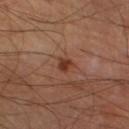Assessment:
This lesion was catalogued during total-body skin photography and was not selected for biopsy.
Clinical summary:
On the leg. A region of skin cropped from a whole-body photographic capture, roughly 15 mm wide. The subject is a male roughly 65 years of age.